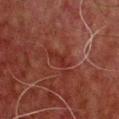follow-up: imaged on a skin check; not biopsied | subject: male, in their 60s | TBP lesion metrics: an area of roughly 4 mm², an eccentricity of roughly 0.9, and a symmetry-axis asymmetry near 0.35 | acquisition: ~15 mm tile from a whole-body skin photo | illumination: cross-polarized | anatomic site: the chest.